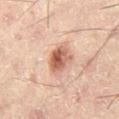biopsy_status: not biopsied; imaged during a skin examination
lighting: cross-polarized
automated_metrics:
  eccentricity: 0.45
  shape_asymmetry: 0.25
  cielab_L: 52
  cielab_a: 22
  cielab_b: 28
  vs_skin_contrast_norm: 9.5
  border_irregularity_0_10: 2.5
  peripheral_color_asymmetry: 2.0
lesion_size:
  long_diameter_mm_approx: 3.0
image:
  source: total-body photography crop
  field_of_view_mm: 15
patient:
  sex: male
  age_approx: 50
site: right thigh